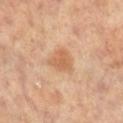Captured during whole-body skin photography for melanoma surveillance; the lesion was not biopsied.
A roughly 15 mm field-of-view crop from a total-body skin photograph.
A female patient, aged approximately 65.
Approximately 3 mm at its widest.
Located on the left leg.
Automated image analysis of the tile measured an area of roughly 5.5 mm² and a symmetry-axis asymmetry near 0.45. And it measured a border-irregularity index near 4/10, a color-variation rating of about 2.5/10, and radial color variation of about 1. And it measured an automated nevus-likeness rating near 65 out of 100 and a detector confidence of about 100 out of 100 that the crop contains a lesion.